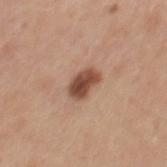Q: Was a biopsy performed?
A: total-body-photography surveillance lesion; no biopsy
Q: What lighting was used for the tile?
A: white-light illumination
Q: What is the lesion's diameter?
A: about 3.5 mm
Q: Where on the body is the lesion?
A: the mid back
Q: How was this image acquired?
A: total-body-photography crop, ~15 mm field of view
Q: Patient demographics?
A: male, roughly 30 years of age